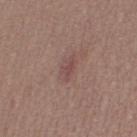  biopsy_status: not biopsied; imaged during a skin examination
  site: leg
  lesion_size:
    long_diameter_mm_approx: 3.0
  lighting: white-light
  image:
    source: total-body photography crop
    field_of_view_mm: 15
  patient:
    sex: female
    age_approx: 20